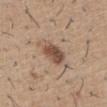Q: What kind of image is this?
A: total-body-photography crop, ~15 mm field of view
Q: Lesion location?
A: the front of the torso
Q: What did automated image analysis measure?
A: a border-irregularity rating of about 1.5/10 and radial color variation of about 1.5
Q: Lesion size?
A: ~3.5 mm (longest diameter)
Q: Patient demographics?
A: male, approximately 60 years of age
Q: Illumination type?
A: white-light illumination